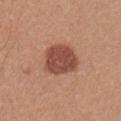notes=no biopsy performed (imaged during a skin exam); size=~4.5 mm (longest diameter); location=the arm; patient=male, aged 33–37; image source=15 mm crop, total-body photography.